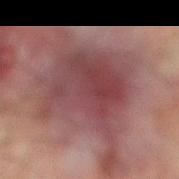Case summary:
– biopsy status — total-body-photography surveillance lesion; no biopsy
– imaging modality — ~15 mm crop, total-body skin-cancer survey
– illumination — cross-polarized illumination
– patient — male, aged approximately 75
– automated metrics — a classifier nevus-likeness of about 5/100 and lesion-presence confidence of about 80/100
– site — the right lower leg